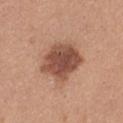Impression:
The lesion was tiled from a total-body skin photograph and was not biopsied.
Image and clinical context:
The subject is a female roughly 35 years of age. The lesion is located on the left thigh. A region of skin cropped from a whole-body photographic capture, roughly 15 mm wide. This is a white-light tile.